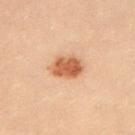From the chest. This image is a 15 mm lesion crop taken from a total-body photograph. The subject is a female in their 20s.